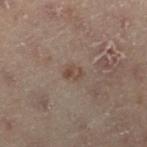Recorded during total-body skin imaging; not selected for excision or biopsy. About 2.5 mm across. A female subject in their mid- to late 50s. A roughly 15 mm field-of-view crop from a total-body skin photograph. This is a cross-polarized tile. Located on the left leg.